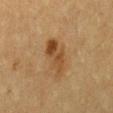biopsy_status: not biopsied; imaged during a skin examination
site: left thigh
lighting: cross-polarized
lesion_size:
  long_diameter_mm_approx: 5.5
patient:
  sex: female
  age_approx: 55
image:
  source: total-body photography crop
  field_of_view_mm: 15
automated_metrics:
  area_mm2_approx: 10.0
  eccentricity: 0.9
  shape_asymmetry: 0.3
  cielab_L: 40
  cielab_a: 17
  cielab_b: 32
  vs_skin_darker_L: 8.0
  vs_skin_contrast_norm: 7.5
  border_irregularity_0_10: 4.0
  color_variation_0_10: 6.0
  peripheral_color_asymmetry: 2.0